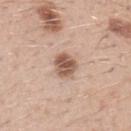Q: What are the patient's age and sex?
A: male, aged around 30
Q: What is the anatomic site?
A: the arm
Q: How was this image acquired?
A: ~15 mm tile from a whole-body skin photo
Q: Automated lesion metrics?
A: a lesion area of about 6.5 mm², an outline eccentricity of about 0.55 (0 = round, 1 = elongated), and a symmetry-axis asymmetry near 0.2; a border-irregularity rating of about 2/10 and a within-lesion color-variation index near 4/10
Q: Lesion size?
A: ≈3 mm
Q: Illumination type?
A: white-light illumination